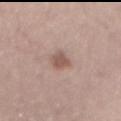Clinical impression: No biopsy was performed on this lesion — it was imaged during a full skin examination and was not determined to be concerning. Acquisition and patient details: The lesion is on the abdomen. A 15 mm close-up extracted from a 3D total-body photography capture. The tile uses white-light illumination. An algorithmic analysis of the crop reported a lesion area of about 5 mm². The software also gave a border-irregularity index near 2.5/10, a within-lesion color-variation index near 2/10, and a peripheral color-asymmetry measure near 0.5. It also reported a classifier nevus-likeness of about 65/100 and a detector confidence of about 100 out of 100 that the crop contains a lesion. Longest diameter approximately 3.5 mm. The patient is a female aged 63 to 67.